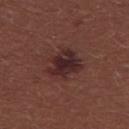Clinical impression: Imaged during a routine full-body skin examination; the lesion was not biopsied and no histopathology is available. Acquisition and patient details: The patient is a female in their mid- to late 20s. This image is a 15 mm lesion crop taken from a total-body photograph. Approximately 4 mm at its widest. The lesion is on the back.